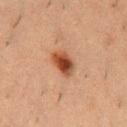biopsy status: no biopsy performed (imaged during a skin exam)
tile lighting: cross-polarized
lesion diameter: ~3.5 mm (longest diameter)
patient: male, about 50 years old
image source: ~15 mm tile from a whole-body skin photo
body site: the chest